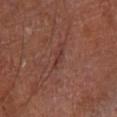Q: Was a biopsy performed?
A: no biopsy performed (imaged during a skin exam)
Q: Illumination type?
A: cross-polarized illumination
Q: Where on the body is the lesion?
A: the leg
Q: What are the patient's age and sex?
A: male, aged approximately 65
Q: How was this image acquired?
A: 15 mm crop, total-body photography